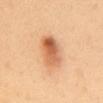Impression:
Captured during whole-body skin photography for melanoma surveillance; the lesion was not biopsied.
Image and clinical context:
A close-up tile cropped from a whole-body skin photograph, about 15 mm across. Automated image analysis of the tile measured roughly 14 lightness units darker than nearby skin. It also reported a border-irregularity index near 2.5/10 and a within-lesion color-variation index near 9/10. A female subject, aged approximately 50. Captured under cross-polarized illumination. Measured at roughly 5.5 mm in maximum diameter. From the back.